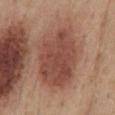Acquisition and patient details:
The subject is a male in their mid-50s. A roughly 15 mm field-of-view crop from a total-body skin photograph. The lesion is located on the mid back.